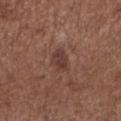Impression:
This lesion was catalogued during total-body skin photography and was not selected for biopsy.
Image and clinical context:
The lesion is located on the left lower leg. A region of skin cropped from a whole-body photographic capture, roughly 15 mm wide. Automated image analysis of the tile measured a mean CIELAB color near L≈37 a*≈20 b*≈23 and a lesion-to-skin contrast of about 7 (normalized; higher = more distinct). And it measured a within-lesion color-variation index near 1.5/10 and a peripheral color-asymmetry measure near 0.5. It also reported an automated nevus-likeness rating near 10 out of 100 and lesion-presence confidence of about 100/100. A female patient in their mid- to late 50s. Longest diameter approximately 2.5 mm. Captured under white-light illumination.